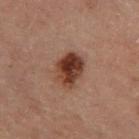Q: What is the imaging modality?
A: total-body-photography crop, ~15 mm field of view
Q: What are the patient's age and sex?
A: male, roughly 70 years of age
Q: What is the lesion's diameter?
A: ~4.5 mm (longest diameter)
Q: Illumination type?
A: cross-polarized
Q: What did automated image analysis measure?
A: a footprint of about 11 mm², an outline eccentricity of about 0.75 (0 = round, 1 = elongated), and a shape-asymmetry score of about 0.15 (0 = symmetric); a border-irregularity rating of about 1.5/10, a color-variation rating of about 7/10, and radial color variation of about 2.5
Q: Lesion location?
A: the left lower leg